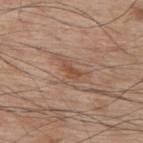The lesion was tiled from a total-body skin photograph and was not biopsied. A male subject, roughly 70 years of age. From the upper back. Measured at roughly 2.5 mm in maximum diameter. The total-body-photography lesion software estimated a lesion color around L≈49 a*≈21 b*≈31 in CIELAB, a lesion–skin lightness drop of about 8, and a normalized lesion–skin contrast near 6.5. The software also gave border irregularity of about 4.5 on a 0–10 scale and a within-lesion color-variation index near 0.5/10. The software also gave a detector confidence of about 95 out of 100 that the crop contains a lesion. Cropped from a whole-body photographic skin survey; the tile spans about 15 mm. Imaged with white-light lighting.